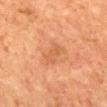biopsy status=no biopsy performed (imaged during a skin exam)
acquisition=15 mm crop, total-body photography
lesion diameter=~3.5 mm (longest diameter)
patient=female, aged approximately 60
location=the mid back
lighting=cross-polarized illumination
TBP lesion metrics=an average lesion color of about L≈48 a*≈23 b*≈33 (CIELAB); an automated nevus-likeness rating near 0 out of 100 and lesion-presence confidence of about 100/100